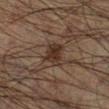Recorded during total-body skin imaging; not selected for excision or biopsy.
A male subject aged 48–52.
Cropped from a whole-body photographic skin survey; the tile spans about 15 mm.
Located on the leg.